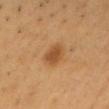<record>
  <biopsy_status>not biopsied; imaged during a skin examination</biopsy_status>
  <lesion_size>
    <long_diameter_mm_approx>3.5</long_diameter_mm_approx>
  </lesion_size>
  <lighting>cross-polarized</lighting>
  <site>chest</site>
  <patient>
    <sex>male</sex>
    <age_approx>60</age_approx>
  </patient>
  <image>
    <source>total-body photography crop</source>
    <field_of_view_mm>15</field_of_view_mm>
  </image>
</record>